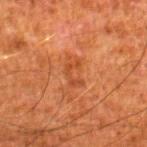| field | value |
|---|---|
| biopsy status | imaged on a skin check; not biopsied |
| illumination | cross-polarized |
| anatomic site | the right lower leg |
| image | total-body-photography crop, ~15 mm field of view |
| size | ≈3 mm |
| subject | male, aged around 80 |